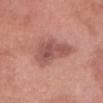notes — total-body-photography surveillance lesion; no biopsy
patient — female, roughly 75 years of age
size — about 5.5 mm
lighting — white-light illumination
image source — total-body-photography crop, ~15 mm field of view
automated metrics — a lesion area of about 15 mm² and a shape eccentricity near 0.75; an average lesion color of about L≈53 a*≈25 b*≈25 (CIELAB), about 10 CIELAB-L* units darker than the surrounding skin, and a normalized lesion–skin contrast near 7; a classifier nevus-likeness of about 15/100 and lesion-presence confidence of about 100/100
site — the head or neck